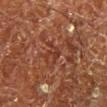{"biopsy_status": "not biopsied; imaged during a skin examination", "automated_metrics": {"cielab_L": 35, "cielab_a": 26, "cielab_b": 29, "vs_skin_darker_L": 6.0, "vs_skin_contrast_norm": 5.5, "border_irregularity_0_10": 5.0, "color_variation_0_10": 0.5, "peripheral_color_asymmetry": 0.0}, "patient": {"sex": "male", "age_approx": 65}, "lesion_size": {"long_diameter_mm_approx": 2.5}, "lighting": "cross-polarized", "image": {"source": "total-body photography crop", "field_of_view_mm": 15}, "site": "right lower leg"}